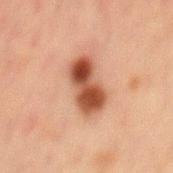Impression:
Recorded during total-body skin imaging; not selected for excision or biopsy.
Acquisition and patient details:
The subject is a male aged around 50. Automated image analysis of the tile measured an area of roughly 11 mm². The analysis additionally found a normalized border contrast of about 12. The analysis additionally found a border-irregularity index near 2.5/10 and radial color variation of about 1.5. It also reported an automated nevus-likeness rating near 85 out of 100 and lesion-presence confidence of about 100/100. Cropped from a total-body skin-imaging series; the visible field is about 15 mm. Imaged with cross-polarized lighting. Located on the mid back. Longest diameter approximately 5.5 mm.